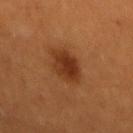biopsy status = total-body-photography surveillance lesion; no biopsy | subject = male, in their 60s | site = the back | acquisition = total-body-photography crop, ~15 mm field of view.